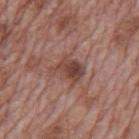<tbp_lesion>
  <biopsy_status>not biopsied; imaged during a skin examination</biopsy_status>
  <automated_metrics>
    <area_mm2_approx>7.0</area_mm2_approx>
    <eccentricity>0.65</eccentricity>
    <shape_asymmetry>0.35</shape_asymmetry>
    <cielab_L>43</cielab_L>
    <cielab_a>21</cielab_a>
    <cielab_b>24</cielab_b>
    <vs_skin_contrast_norm>8.0</vs_skin_contrast_norm>
    <border_irregularity_0_10>3.5</border_irregularity_0_10>
    <color_variation_0_10>6.0</color_variation_0_10>
    <peripheral_color_asymmetry>2.0</peripheral_color_asymmetry>
    <nevus_likeness_0_100>20</nevus_likeness_0_100>
    <lesion_detection_confidence_0_100>100</lesion_detection_confidence_0_100>
  </automated_metrics>
  <lighting>white-light</lighting>
  <lesion_size>
    <long_diameter_mm_approx>3.5</long_diameter_mm_approx>
  </lesion_size>
  <patient>
    <sex>male</sex>
    <age_approx>70</age_approx>
  </patient>
  <image>
    <source>total-body photography crop</source>
    <field_of_view_mm>15</field_of_view_mm>
  </image>
  <site>back</site>
</tbp_lesion>